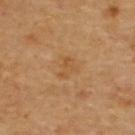biopsy status: no biopsy performed (imaged during a skin exam) | body site: the upper back | diameter: ≈2.5 mm | image source: total-body-photography crop, ~15 mm field of view | illumination: cross-polarized illumination | patient: aged approximately 60.